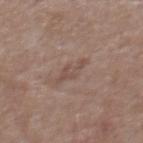follow-up: imaged on a skin check; not biopsied
TBP lesion metrics: a border-irregularity rating of about 6/10, internal color variation of about 0 on a 0–10 scale, and peripheral color asymmetry of about 0; a classifier nevus-likeness of about 0/100 and lesion-presence confidence of about 100/100
subject: male, aged 53–57
size: about 3.5 mm
location: the chest
imaging modality: ~15 mm tile from a whole-body skin photo